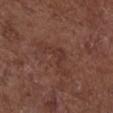workup=no biopsy performed (imaged during a skin exam) | image=total-body-photography crop, ~15 mm field of view | lighting=white-light | anatomic site=the front of the torso | diameter=≈6 mm | patient=female, roughly 80 years of age.